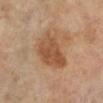The lesion was photographed on a routine skin check and not biopsied; there is no pathology result. On the left lower leg. A roughly 15 mm field-of-view crop from a total-body skin photograph. The subject is a female in their 70s.